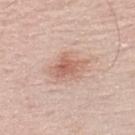The lesion was tiled from a total-body skin photograph and was not biopsied. A male patient, aged 68 to 72. Located on the left lower leg. About 4 mm across. Imaged with white-light lighting. An algorithmic analysis of the crop reported a classifier nevus-likeness of about 30/100 and a lesion-detection confidence of about 100/100. A 15 mm close-up extracted from a 3D total-body photography capture.